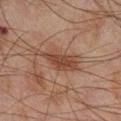notes=catalogued during a skin exam; not biopsied
image source=~15 mm tile from a whole-body skin photo
subject=male, approximately 45 years of age
size=~5.5 mm (longest diameter)
body site=the right lower leg